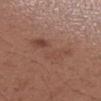Q: How was this image acquired?
A: 15 mm crop, total-body photography
Q: Where on the body is the lesion?
A: the right forearm
Q: Patient demographics?
A: female, aged approximately 30